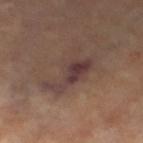Q: Was this lesion biopsied?
A: total-body-photography surveillance lesion; no biopsy
Q: How was the tile lit?
A: cross-polarized illumination
Q: How was this image acquired?
A: ~15 mm crop, total-body skin-cancer survey
Q: Lesion size?
A: ≈6.5 mm
Q: What did automated image analysis measure?
A: a footprint of about 13 mm², a shape eccentricity near 0.9, and a symmetry-axis asymmetry near 0.55; a mean CIELAB color near L≈39 a*≈17 b*≈19, about 8 CIELAB-L* units darker than the surrounding skin, and a normalized border contrast of about 8.5; an automated nevus-likeness rating near 5 out of 100 and a lesion-detection confidence of about 70/100
Q: Where on the body is the lesion?
A: the leg
Q: Who is the patient?
A: female, aged 63–67